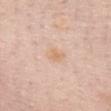  lighting: white-light
  site: front of the torso
  patient:
    sex: female
    age_approx: 60
  lesion_size:
    long_diameter_mm_approx: 2.5
  image:
    source: total-body photography crop
    field_of_view_mm: 15